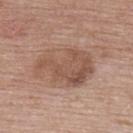{
  "biopsy_status": "not biopsied; imaged during a skin examination",
  "lighting": "white-light",
  "lesion_size": {
    "long_diameter_mm_approx": 7.0
  },
  "patient": {
    "sex": "female",
    "age_approx": 50
  },
  "image": {
    "source": "total-body photography crop",
    "field_of_view_mm": 15
  },
  "automated_metrics": {
    "nevus_likeness_0_100": 5,
    "lesion_detection_confidence_0_100": 100
  },
  "site": "upper back"
}